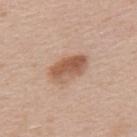The lesion was photographed on a routine skin check and not biopsied; there is no pathology result. A roughly 15 mm field-of-view crop from a total-body skin photograph. Captured under white-light illumination. From the back. The subject is a male in their mid- to late 30s. About 4.5 mm across.